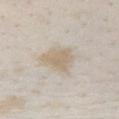Impression: This lesion was catalogued during total-body skin photography and was not selected for biopsy. Background: Longest diameter approximately 4 mm. A female patient, aged approximately 25. The tile uses white-light illumination. The lesion is located on the chest. Automated tile analysis of the lesion measured a lesion–skin lightness drop of about 8 and a normalized lesion–skin contrast near 6.5. The analysis additionally found a border-irregularity index near 2.5/10, a within-lesion color-variation index near 2/10, and radial color variation of about 0.5. And it measured a nevus-likeness score of about 0/100 and a lesion-detection confidence of about 55/100. Cropped from a whole-body photographic skin survey; the tile spans about 15 mm.